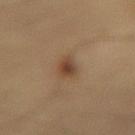Impression:
The lesion was photographed on a routine skin check and not biopsied; there is no pathology result.
Context:
A male subject, in their 60s. The tile uses cross-polarized illumination. About 3.5 mm across. A 15 mm crop from a total-body photograph taken for skin-cancer surveillance. The lesion is on the lower back.